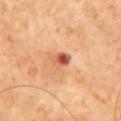Case summary:
• follow-up · imaged on a skin check; not biopsied
• subject · male, about 65 years old
• automated metrics · an eccentricity of roughly 0.7 and two-axis asymmetry of about 0.35; a lesion color around L≈57 a*≈29 b*≈35 in CIELAB, a lesion–skin lightness drop of about 13, and a lesion-to-skin contrast of about 8.5 (normalized; higher = more distinct); a nevus-likeness score of about 0/100 and a lesion-detection confidence of about 100/100
• image · total-body-photography crop, ~15 mm field of view
• tile lighting · cross-polarized illumination